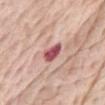Findings:
- subject: male, in their mid- to late 80s or older
- image-analysis metrics: a border-irregularity index near 2.5/10, internal color variation of about 3 on a 0–10 scale, and peripheral color asymmetry of about 1
- diameter: ≈3 mm
- anatomic site: the chest
- imaging modality: 15 mm crop, total-body photography